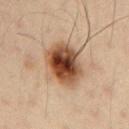Image and clinical context:
This is a cross-polarized tile. On the right upper arm. The patient is a male aged around 35. The lesion-visualizer software estimated a shape eccentricity near 0.55. The analysis additionally found a classifier nevus-likeness of about 100/100 and a detector confidence of about 100 out of 100 that the crop contains a lesion. A 15 mm close-up tile from a total-body photography series done for melanoma screening.